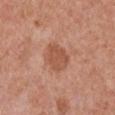Notes:
– biopsy status · no biopsy performed (imaged during a skin exam)
– image · 15 mm crop, total-body photography
– subject · female, roughly 70 years of age
– automated metrics · a mean CIELAB color near L≈53 a*≈25 b*≈32, roughly 9 lightness units darker than nearby skin, and a lesion-to-skin contrast of about 7 (normalized; higher = more distinct)
– tile lighting · white-light
– size · ≈3.5 mm
– location · the chest